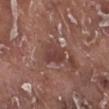Clinical impression:
The lesion was tiled from a total-body skin photograph and was not biopsied.
Background:
The lesion is on the right lower leg. The subject is a male approximately 75 years of age. Measured at roughly 3 mm in maximum diameter. A region of skin cropped from a whole-body photographic capture, roughly 15 mm wide. The tile uses white-light illumination.